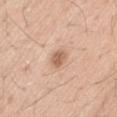biopsy_status: not biopsied; imaged during a skin examination